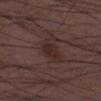workup: catalogued during a skin exam; not biopsied | image source: 15 mm crop, total-body photography | body site: the leg | tile lighting: white-light illumination | subject: male, approximately 50 years of age | automated metrics: a lesion color around L≈25 a*≈18 b*≈17 in CIELAB, a lesion–skin lightness drop of about 6, and a lesion-to-skin contrast of about 7 (normalized; higher = more distinct); border irregularity of about 2.5 on a 0–10 scale, internal color variation of about 1.5 on a 0–10 scale, and peripheral color asymmetry of about 0.5 | diameter: about 2.5 mm.